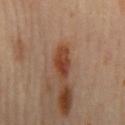Q: Is there a histopathology result?
A: catalogued during a skin exam; not biopsied
Q: What did automated image analysis measure?
A: a footprint of about 6.5 mm², an eccentricity of roughly 0.9, and two-axis asymmetry of about 0.25; a lesion–skin lightness drop of about 12 and a lesion-to-skin contrast of about 10 (normalized; higher = more distinct); border irregularity of about 3 on a 0–10 scale, a color-variation rating of about 4.5/10, and peripheral color asymmetry of about 1.5; a nevus-likeness score of about 90/100 and lesion-presence confidence of about 100/100
Q: Patient demographics?
A: male, aged around 65
Q: How large is the lesion?
A: ≈4.5 mm
Q: What is the anatomic site?
A: the mid back
Q: What is the imaging modality?
A: ~15 mm tile from a whole-body skin photo